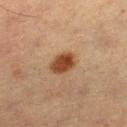- notes: catalogued during a skin exam; not biopsied
- size: ~3.5 mm (longest diameter)
- patient: female, about 55 years old
- site: the right lower leg
- image source: ~15 mm crop, total-body skin-cancer survey